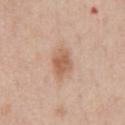Captured during whole-body skin photography for melanoma surveillance; the lesion was not biopsied.